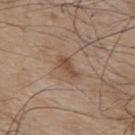Assessment: The lesion was tiled from a total-body skin photograph and was not biopsied. Acquisition and patient details: Captured under white-light illumination. The recorded lesion diameter is about 3 mm. On the mid back. A male patient in their mid- to late 70s. Automated image analysis of the tile measured a border-irregularity index near 3.5/10 and a color-variation rating of about 1/10. Cropped from a whole-body photographic skin survey; the tile spans about 15 mm.